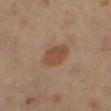Located on the leg. Cropped from a whole-body photographic skin survey; the tile spans about 15 mm. A male patient aged around 60. The tile uses cross-polarized illumination. About 4 mm across.